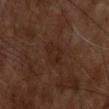notes: catalogued during a skin exam; not biopsied | acquisition: ~15 mm crop, total-body skin-cancer survey | subject: male, in their mid- to late 50s | site: the upper back.